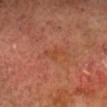| key | value |
|---|---|
| biopsy status | total-body-photography surveillance lesion; no biopsy |
| image source | ~15 mm tile from a whole-body skin photo |
| lesion diameter | ~2.5 mm (longest diameter) |
| site | the head or neck |
| TBP lesion metrics | a border-irregularity index near 4.5/10, a color-variation rating of about 0/10, and a peripheral color-asymmetry measure near 0 |
| subject | male, about 70 years old |
| lighting | cross-polarized illumination |